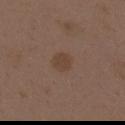No biopsy was performed on this lesion — it was imaged during a full skin examination and was not determined to be concerning.
The lesion's longest dimension is about 2.5 mm.
A 15 mm close-up extracted from a 3D total-body photography capture.
The lesion is on the upper back.
Automated image analysis of the tile measured an outline eccentricity of about 0.55 (0 = round, 1 = elongated). The analysis additionally found a nevus-likeness score of about 40/100 and a detector confidence of about 100 out of 100 that the crop contains a lesion.
The patient is a female aged around 35.
The tile uses white-light illumination.